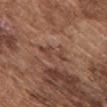{"biopsy_status": "not biopsied; imaged during a skin examination", "image": {"source": "total-body photography crop", "field_of_view_mm": 15}, "site": "chest", "lesion_size": {"long_diameter_mm_approx": 3.5}, "patient": {"sex": "male", "age_approx": 75}}